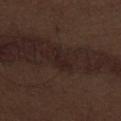biopsy status — catalogued during a skin exam; not biopsied
imaging modality — total-body-photography crop, ~15 mm field of view
body site — the abdomen
subject — male, aged around 70